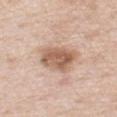| key | value |
|---|---|
| acquisition | total-body-photography crop, ~15 mm field of view |
| tile lighting | white-light |
| patient | male, aged approximately 50 |
| lesion size | ≈4.5 mm |
| body site | the chest |